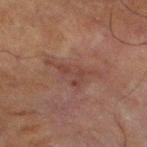workup — catalogued during a skin exam; not biopsied
acquisition — ~15 mm tile from a whole-body skin photo
diameter — ~6 mm (longest diameter)
automated lesion analysis — a lesion area of about 8.5 mm², an outline eccentricity of about 0.85 (0 = round, 1 = elongated), and a shape-asymmetry score of about 0.55 (0 = symmetric); an automated nevus-likeness rating near 0 out of 100
site — the left lower leg
patient — male, approximately 70 years of age
lighting — cross-polarized illumination